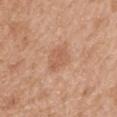patient=female, aged around 40 | image source=total-body-photography crop, ~15 mm field of view | body site=the mid back | lighting=white-light illumination | lesion size=≈3 mm.